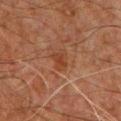Assessment:
Recorded during total-body skin imaging; not selected for excision or biopsy.
Background:
The recorded lesion diameter is about 2.5 mm. A male patient, approximately 60 years of age. From the chest. Imaged with cross-polarized lighting. This image is a 15 mm lesion crop taken from a total-body photograph.